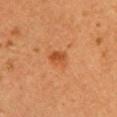{"biopsy_status": "not biopsied; imaged during a skin examination", "image": {"source": "total-body photography crop", "field_of_view_mm": 15}, "automated_metrics": {"cielab_L": 48, "cielab_a": 27, "cielab_b": 40, "vs_skin_darker_L": 9.0, "vs_skin_contrast_norm": 7.0, "nevus_likeness_0_100": 85, "lesion_detection_confidence_0_100": 100}, "patient": {"sex": "female", "age_approx": 30}, "lesion_size": {"long_diameter_mm_approx": 2.5}, "site": "chest", "lighting": "cross-polarized"}